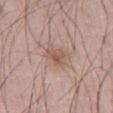Q: Was this lesion biopsied?
A: total-body-photography surveillance lesion; no biopsy
Q: What are the patient's age and sex?
A: male, in their 50s
Q: What is the anatomic site?
A: the abdomen
Q: How was this image acquired?
A: 15 mm crop, total-body photography
Q: Automated lesion metrics?
A: an outline eccentricity of about 0.65 (0 = round, 1 = elongated) and a shape-asymmetry score of about 0.35 (0 = symmetric)
Q: What is the lesion's diameter?
A: ≈2.5 mm